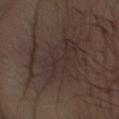– biopsy status — total-body-photography surveillance lesion; no biopsy
– subject — male, aged approximately 50
– diameter — about 9 mm
– automated lesion analysis — a lesion area of about 32 mm², an outline eccentricity of about 0.75 (0 = round, 1 = elongated), and a shape-asymmetry score of about 0.45 (0 = symmetric); a mean CIELAB color near L≈30 a*≈12 b*≈17, a lesion–skin lightness drop of about 5, and a lesion-to-skin contrast of about 5.5 (normalized; higher = more distinct); border irregularity of about 8.5 on a 0–10 scale
– image — ~15 mm crop, total-body skin-cancer survey
– site — the right forearm
– lighting — white-light illumination